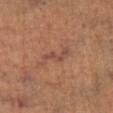Assessment:
Recorded during total-body skin imaging; not selected for excision or biopsy.
Acquisition and patient details:
Approximately 4 mm at its widest. A female patient roughly 65 years of age. Imaged with cross-polarized lighting. Located on the right lower leg. The lesion-visualizer software estimated an eccentricity of roughly 0.9 and a shape-asymmetry score of about 0.6 (0 = symmetric). It also reported a nevus-likeness score of about 0/100 and a detector confidence of about 90 out of 100 that the crop contains a lesion. A lesion tile, about 15 mm wide, cut from a 3D total-body photograph.